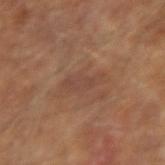acquisition: ~15 mm crop, total-body skin-cancer survey | subject: male, aged 63 to 67 | automated lesion analysis: a footprint of about 6.5 mm² and two-axis asymmetry of about 0.3; a mean CIELAB color near L≈43 a*≈19 b*≈27, about 5 CIELAB-L* units darker than the surrounding skin, and a lesion-to-skin contrast of about 4.5 (normalized; higher = more distinct); a border-irregularity rating of about 4/10 | illumination: cross-polarized | location: the right upper arm.